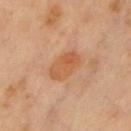Part of a total-body skin-imaging series; this lesion was reviewed on a skin check and was not flagged for biopsy.
Imaged with cross-polarized lighting.
The lesion is on the chest.
This image is a 15 mm lesion crop taken from a total-body photograph.
A male patient aged 53–57.
Measured at roughly 4 mm in maximum diameter.